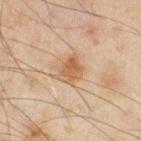Part of a total-body skin-imaging series; this lesion was reviewed on a skin check and was not flagged for biopsy.
Imaged with cross-polarized lighting.
Cropped from a whole-body photographic skin survey; the tile spans about 15 mm.
A male subject, in their mid-40s.
Located on the right thigh.